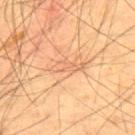{"patient": {"sex": "male", "age_approx": 55}, "site": "upper back", "image": {"source": "total-body photography crop", "field_of_view_mm": 15}}